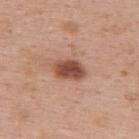workup=catalogued during a skin exam; not biopsied | TBP lesion metrics=border irregularity of about 2 on a 0–10 scale, internal color variation of about 4 on a 0–10 scale, and a peripheral color-asymmetry measure near 1; a detector confidence of about 100 out of 100 that the crop contains a lesion | image=total-body-photography crop, ~15 mm field of view | size=about 4 mm | illumination=white-light | subject=female, aged around 50 | site=the back.